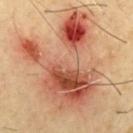No biopsy was performed on this lesion — it was imaged during a full skin examination and was not determined to be concerning.